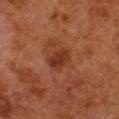Assessment: No biopsy was performed on this lesion — it was imaged during a full skin examination and was not determined to be concerning. Context: On the right lower leg. The subject is a male in their 80s. Longest diameter approximately 3 mm. The tile uses cross-polarized illumination. A region of skin cropped from a whole-body photographic capture, roughly 15 mm wide.